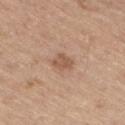Findings:
* lighting — white-light
* diameter — ≈2.5 mm
* image source — total-body-photography crop, ~15 mm field of view
* automated metrics — an average lesion color of about L≈55 a*≈19 b*≈31 (CIELAB), about 9 CIELAB-L* units darker than the surrounding skin, and a normalized lesion–skin contrast near 6.5; a nevus-likeness score of about 15/100
* patient — male, approximately 70 years of age
* location — the right thigh